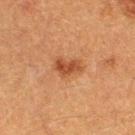Assessment: This lesion was catalogued during total-body skin photography and was not selected for biopsy. Image and clinical context: On the right thigh. Longest diameter approximately 3 mm. The subject is a female aged 38–42. A close-up tile cropped from a whole-body skin photograph, about 15 mm across.